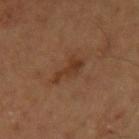Imaged during a routine full-body skin examination; the lesion was not biopsied and no histopathology is available.
A male subject aged 58–62.
The lesion is located on the left upper arm.
A 15 mm close-up tile from a total-body photography series done for melanoma screening.